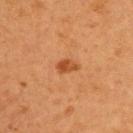No biopsy was performed on this lesion — it was imaged during a full skin examination and was not determined to be concerning.